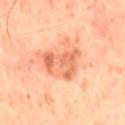Recorded during total-body skin imaging; not selected for excision or biopsy.